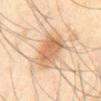Part of a total-body skin-imaging series; this lesion was reviewed on a skin check and was not flagged for biopsy. Located on the abdomen. This is a cross-polarized tile. This image is a 15 mm lesion crop taken from a total-body photograph. Approximately 5.5 mm at its widest. The subject is a male in their mid- to late 40s. Automated image analysis of the tile measured a lesion area of about 17 mm² and an eccentricity of roughly 0.8. The analysis additionally found a border-irregularity index near 3/10 and peripheral color asymmetry of about 1.5.